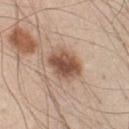The lesion was photographed on a routine skin check and not biopsied; there is no pathology result. An algorithmic analysis of the crop reported an area of roughly 11 mm². And it measured a lesion color around L≈52 a*≈19 b*≈29 in CIELAB, roughly 15 lightness units darker than nearby skin, and a normalized lesion–skin contrast near 10. The software also gave a border-irregularity index near 1.5/10, a color-variation rating of about 6/10, and radial color variation of about 2. And it measured lesion-presence confidence of about 100/100. The subject is a male about 45 years old. This is a white-light tile. The lesion's longest dimension is about 4 mm. Cropped from a total-body skin-imaging series; the visible field is about 15 mm. From the front of the torso.